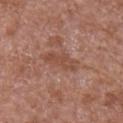biopsy status: catalogued during a skin exam; not biopsied
location: the right upper arm
image: total-body-photography crop, ~15 mm field of view
illumination: white-light illumination
size: ≈4.5 mm
automated metrics: a lesion area of about 6.5 mm², a shape eccentricity near 0.95, and a shape-asymmetry score of about 0.25 (0 = symmetric); about 7 CIELAB-L* units darker than the surrounding skin and a lesion-to-skin contrast of about 6 (normalized; higher = more distinct)
subject: male, approximately 65 years of age